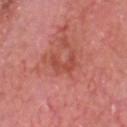Q: What is the lesion's diameter?
A: ~4 mm (longest diameter)
Q: How was this image acquired?
A: total-body-photography crop, ~15 mm field of view
Q: Automated lesion metrics?
A: about 7 CIELAB-L* units darker than the surrounding skin and a normalized lesion–skin contrast near 6; a classifier nevus-likeness of about 0/100 and lesion-presence confidence of about 95/100
Q: Who is the patient?
A: male, roughly 70 years of age
Q: Where on the body is the lesion?
A: the head or neck